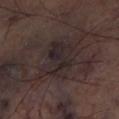Clinical impression:
The lesion was tiled from a total-body skin photograph and was not biopsied.
Clinical summary:
Approximately 5 mm at its widest. Located on the leg. This image is a 15 mm lesion crop taken from a total-body photograph. The lesion-visualizer software estimated a border-irregularity index near 3.5/10, a color-variation rating of about 4.5/10, and peripheral color asymmetry of about 1.5. The analysis additionally found a nevus-likeness score of about 0/100 and a lesion-detection confidence of about 90/100. Imaged with cross-polarized lighting.